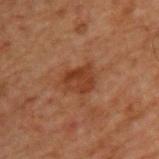Part of a total-body skin-imaging series; this lesion was reviewed on a skin check and was not flagged for biopsy. The recorded lesion diameter is about 3 mm. On the back. A male patient, in their 70s. A 15 mm close-up tile from a total-body photography series done for melanoma screening.